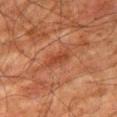site: leg
automated_metrics:
  area_mm2_approx: 4.0
  eccentricity: 0.9
  border_irregularity_0_10: 3.0
  color_variation_0_10: 2.0
  peripheral_color_asymmetry: 0.5
  nevus_likeness_0_100: 0
  lesion_detection_confidence_0_100: 95
patient:
  sex: male
  age_approx: 80
lesion_size:
  long_diameter_mm_approx: 3.5
lighting: cross-polarized
image:
  source: total-body photography crop
  field_of_view_mm: 15